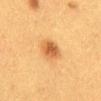notes: no biopsy performed (imaged during a skin exam)
patient: female, in their 40s
lighting: cross-polarized
image: total-body-photography crop, ~15 mm field of view
TBP lesion metrics: a footprint of about 6.5 mm², an outline eccentricity of about 0.4 (0 = round, 1 = elongated), and a shape-asymmetry score of about 0.2 (0 = symmetric); a classifier nevus-likeness of about 100/100 and a lesion-detection confidence of about 100/100
anatomic site: the abdomen
lesion size: ≈3 mm